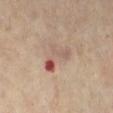Part of a total-body skin-imaging series; this lesion was reviewed on a skin check and was not flagged for biopsy. Longest diameter approximately 4.5 mm. This image is a 15 mm lesion crop taken from a total-body photograph. The lesion is on the right lower leg. The subject is a female aged around 60.